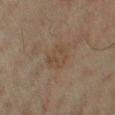image=total-body-photography crop, ~15 mm field of view; patient=male, roughly 65 years of age; anatomic site=the leg.